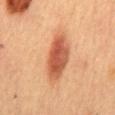On the mid back. The patient is a female aged 58 to 62. About 7 mm across. Imaged with cross-polarized lighting. A 15 mm close-up tile from a total-body photography series done for melanoma screening.